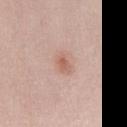Clinical impression:
The lesion was tiled from a total-body skin photograph and was not biopsied.
Image and clinical context:
The recorded lesion diameter is about 3 mm. A female patient approximately 40 years of age. Cropped from a whole-body photographic skin survey; the tile spans about 15 mm. Located on the front of the torso. Automated image analysis of the tile measured an automated nevus-likeness rating near 45 out of 100 and lesion-presence confidence of about 100/100. The tile uses white-light illumination.